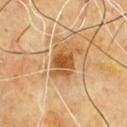Assessment:
No biopsy was performed on this lesion — it was imaged during a full skin examination and was not determined to be concerning.
Clinical summary:
A roughly 15 mm field-of-view crop from a total-body skin photograph. Approximately 4 mm at its widest. An algorithmic analysis of the crop reported a footprint of about 9 mm² and an eccentricity of roughly 0.7. The software also gave a within-lesion color-variation index near 5.5/10 and peripheral color asymmetry of about 1.5. A male patient about 65 years old. The lesion is located on the front of the torso.